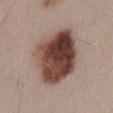Case summary:
• biopsy status: total-body-photography surveillance lesion; no biopsy
• location: the lower back
• acquisition: ~15 mm tile from a whole-body skin photo
• size: ~7 mm (longest diameter)
• patient: male, aged 53 to 57
• automated metrics: a border-irregularity index near 1.5/10 and a within-lesion color-variation index near 9.5/10; a classifier nevus-likeness of about 85/100 and a detector confidence of about 100 out of 100 that the crop contains a lesion
• illumination: white-light